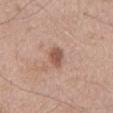Findings:
* biopsy status: imaged on a skin check; not biopsied
* lesion diameter: about 3 mm
* anatomic site: the mid back
* automated lesion analysis: a footprint of about 4 mm²; a border-irregularity index near 2/10, internal color variation of about 3 on a 0–10 scale, and radial color variation of about 1
* image source: ~15 mm crop, total-body skin-cancer survey
* patient: male, about 50 years old
* lighting: white-light